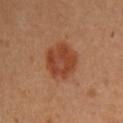The lesion was photographed on a routine skin check and not biopsied; there is no pathology result. A female subject, approximately 30 years of age. A 15 mm close-up extracted from a 3D total-body photography capture. Automated image analysis of the tile measured a normalized border contrast of about 8. And it measured a nevus-likeness score of about 95/100 and a detector confidence of about 100 out of 100 that the crop contains a lesion. Imaged with cross-polarized lighting. The lesion is on the right upper arm.